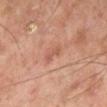A lesion tile, about 15 mm wide, cut from a 3D total-body photograph.
Captured under cross-polarized illumination.
A male patient, aged around 50.
From the left lower leg.
Approximately 2.5 mm at its widest.